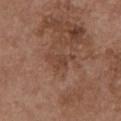workup: catalogued during a skin exam; not biopsied | patient: female, aged around 65 | illumination: white-light | site: the chest | TBP lesion metrics: a lesion area of about 6 mm², an outline eccentricity of about 0.75 (0 = round, 1 = elongated), and a symmetry-axis asymmetry near 0.55; an automated nevus-likeness rating near 0 out of 100 and lesion-presence confidence of about 100/100 | image source: total-body-photography crop, ~15 mm field of view.